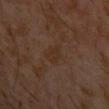automated lesion analysis=an area of roughly 4.5 mm² and two-axis asymmetry of about 0.35; roughly 4 lightness units darker than nearby skin and a normalized border contrast of about 5.5 | site=the left upper arm | patient=male, aged approximately 30 | imaging modality=15 mm crop, total-body photography.